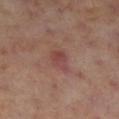About 2.5 mm across. The patient is a female in their mid-50s. Automated tile analysis of the lesion measured a border-irregularity rating of about 2.5/10, a color-variation rating of about 3.5/10, and peripheral color asymmetry of about 1.5. It also reported a nevus-likeness score of about 0/100 and lesion-presence confidence of about 100/100. From the right lower leg. A 15 mm crop from a total-body photograph taken for skin-cancer surveillance. The tile uses cross-polarized illumination.